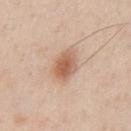Findings:
• notes · total-body-photography surveillance lesion; no biopsy
• patient · male, aged 38–42
• anatomic site · the chest
• lesion size · ≈3.5 mm
• illumination · white-light
• TBP lesion metrics · a border-irregularity index near 1.5/10, a within-lesion color-variation index near 5.5/10, and radial color variation of about 1.5
• acquisition · ~15 mm crop, total-body skin-cancer survey